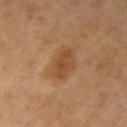biopsy status: imaged on a skin check; not biopsied | imaging modality: 15 mm crop, total-body photography | diameter: about 4 mm | subject: female, aged 63–67 | location: the left arm.